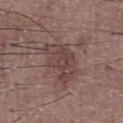Clinical impression: The lesion was tiled from a total-body skin photograph and was not biopsied. Acquisition and patient details: Approximately 5.5 mm at its widest. This is a white-light tile. This image is a 15 mm lesion crop taken from a total-body photograph. The lesion is located on the chest. A male patient, aged approximately 60.